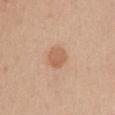– biopsy status · no biopsy performed (imaged during a skin exam)
– site · the chest
– image source · 15 mm crop, total-body photography
– patient · female, roughly 40 years of age